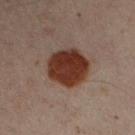The lesion was photographed on a routine skin check and not biopsied; there is no pathology result.
The lesion is located on the right upper arm.
Longest diameter approximately 5 mm.
The subject is a male aged around 50.
The total-body-photography lesion software estimated an average lesion color of about L≈29 a*≈20 b*≈24 (CIELAB). The software also gave border irregularity of about 1.5 on a 0–10 scale and peripheral color asymmetry of about 1. It also reported an automated nevus-likeness rating near 100 out of 100.
A region of skin cropped from a whole-body photographic capture, roughly 15 mm wide.
Imaged with cross-polarized lighting.